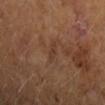Notes:
– follow-up · imaged on a skin check; not biopsied
– patient · female, aged 58 to 62
– TBP lesion metrics · a footprint of about 2.5 mm² and a shape-asymmetry score of about 0.3 (0 = symmetric); a nevus-likeness score of about 0/100 and a detector confidence of about 95 out of 100 that the crop contains a lesion
– body site · the right forearm
– lesion size · about 2.5 mm
– image source · ~15 mm tile from a whole-body skin photo
– lighting · cross-polarized